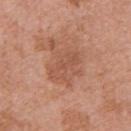workup — catalogued during a skin exam; not biopsied.